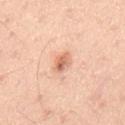<record>
<biopsy_status>not biopsied; imaged during a skin examination</biopsy_status>
<patient>
  <sex>male</sex>
  <age_approx>45</age_approx>
</patient>
<image>
  <source>total-body photography crop</source>
  <field_of_view_mm>15</field_of_view_mm>
</image>
<site>mid back</site>
<lighting>white-light</lighting>
<lesion_size>
  <long_diameter_mm_approx>2.5</long_diameter_mm_approx>
</lesion_size>
</record>